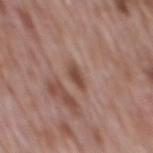This lesion was catalogued during total-body skin photography and was not selected for biopsy.
A close-up tile cropped from a whole-body skin photograph, about 15 mm across.
The lesion-visualizer software estimated an area of roughly 3.5 mm², a shape eccentricity near 0.85, and a symmetry-axis asymmetry near 0.35. The analysis additionally found a border-irregularity index near 3/10, a within-lesion color-variation index near 1.5/10, and peripheral color asymmetry of about 0.5.
About 3 mm across.
A male subject about 70 years old.
From the mid back.
Captured under white-light illumination.